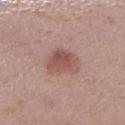Part of a total-body skin-imaging series; this lesion was reviewed on a skin check and was not flagged for biopsy. Imaged with white-light lighting. A close-up tile cropped from a whole-body skin photograph, about 15 mm across. On the right lower leg. A female subject approximately 30 years of age.